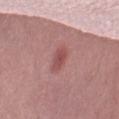Findings:
* automated lesion analysis — a footprint of about 3.5 mm² and a shape-asymmetry score of about 0.25 (0 = symmetric); a border-irregularity rating of about 2.5/10, internal color variation of about 1.5 on a 0–10 scale, and radial color variation of about 0.5; a nevus-likeness score of about 55/100
* subject — female, in their mid-50s
* lesion size — ~3 mm (longest diameter)
* image source — ~15 mm crop, total-body skin-cancer survey
* lighting — white-light illumination
* site — the lower back